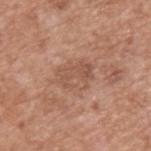Assessment:
Part of a total-body skin-imaging series; this lesion was reviewed on a skin check and was not flagged for biopsy.
Context:
A male patient roughly 65 years of age. Imaged with white-light lighting. A 15 mm close-up extracted from a 3D total-body photography capture. An algorithmic analysis of the crop reported about 7 CIELAB-L* units darker than the surrounding skin and a lesion-to-skin contrast of about 5 (normalized; higher = more distinct). And it measured a border-irregularity index near 3/10, a within-lesion color-variation index near 3.5/10, and a peripheral color-asymmetry measure near 1.5. And it measured an automated nevus-likeness rating near 0 out of 100 and a lesion-detection confidence of about 100/100. Longest diameter approximately 4 mm. From the upper back.